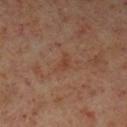Notes:
– follow-up · no biopsy performed (imaged during a skin exam)
– imaging modality · total-body-photography crop, ~15 mm field of view
– site · the left lower leg
– patient · male, approximately 60 years of age
– diameter · ≈3 mm
– lighting · cross-polarized